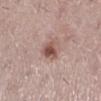Q: Was a biopsy performed?
A: no biopsy performed (imaged during a skin exam)
Q: What kind of image is this?
A: 15 mm crop, total-body photography
Q: Lesion location?
A: the right lower leg
Q: What did automated image analysis measure?
A: a footprint of about 4.5 mm², an outline eccentricity of about 0.6 (0 = round, 1 = elongated), and a symmetry-axis asymmetry near 0.3; a mean CIELAB color near L≈51 a*≈21 b*≈24, a lesion–skin lightness drop of about 13, and a normalized lesion–skin contrast near 9; a color-variation rating of about 3.5/10 and peripheral color asymmetry of about 1
Q: How was the tile lit?
A: white-light illumination
Q: What are the patient's age and sex?
A: female, aged 38 to 42